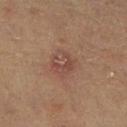Recorded during total-body skin imaging; not selected for excision or biopsy. The lesion-visualizer software estimated a lesion color around L≈39 a*≈17 b*≈22 in CIELAB, a lesion–skin lightness drop of about 6, and a lesion-to-skin contrast of about 5.5 (normalized; higher = more distinct). And it measured a nevus-likeness score of about 5/100 and a lesion-detection confidence of about 100/100. The tile uses cross-polarized illumination. The subject is a male aged 83 or older. A roughly 15 mm field-of-view crop from a total-body skin photograph. On the leg. About 3.5 mm across.